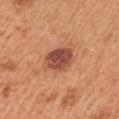Case summary:
- notes: total-body-photography surveillance lesion; no biopsy
- site: the chest
- patient: male, aged around 55
- image source: ~15 mm crop, total-body skin-cancer survey
- size: ~4 mm (longest diameter)
- automated metrics: a mean CIELAB color near L≈49 a*≈27 b*≈30, about 14 CIELAB-L* units darker than the surrounding skin, and a lesion-to-skin contrast of about 10.5 (normalized; higher = more distinct)